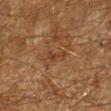Clinical impression: Captured during whole-body skin photography for melanoma surveillance; the lesion was not biopsied. Background: The recorded lesion diameter is about 2.5 mm. A male patient aged approximately 60. A close-up tile cropped from a whole-body skin photograph, about 15 mm across. The lesion is located on the left lower leg.